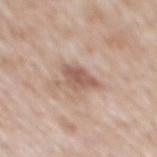Q: Was this lesion biopsied?
A: no biopsy performed (imaged during a skin exam)
Q: Patient demographics?
A: male, aged around 50
Q: What kind of image is this?
A: total-body-photography crop, ~15 mm field of view
Q: How large is the lesion?
A: about 3.5 mm
Q: Where on the body is the lesion?
A: the mid back
Q: Illumination type?
A: white-light